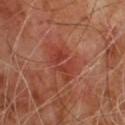| field | value |
|---|---|
| notes | no biopsy performed (imaged during a skin exam) |
| lesion diameter | ≈5 mm |
| image | ~15 mm crop, total-body skin-cancer survey |
| image-analysis metrics | a lesion area of about 9.5 mm² and an eccentricity of roughly 0.8 |
| subject | male, in their mid-60s |
| illumination | cross-polarized |